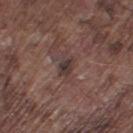notes: imaged on a skin check; not biopsied | subject: male, approximately 75 years of age | illumination: white-light | image source: ~15 mm crop, total-body skin-cancer survey | anatomic site: the leg.